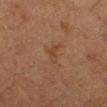Clinical summary:
Approximately 3 mm at its widest. A female patient aged around 50. Automated image analysis of the tile measured an automated nevus-likeness rating near 0 out of 100 and a detector confidence of about 100 out of 100 that the crop contains a lesion. Located on the left lower leg. A 15 mm close-up extracted from a 3D total-body photography capture.